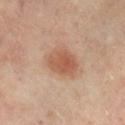This lesion was catalogued during total-body skin photography and was not selected for biopsy. A 15 mm close-up extracted from a 3D total-body photography capture. The lesion is on the leg. An algorithmic analysis of the crop reported an eccentricity of roughly 0.6 and two-axis asymmetry of about 0.15. It also reported border irregularity of about 2 on a 0–10 scale, internal color variation of about 3.5 on a 0–10 scale, and peripheral color asymmetry of about 1. The tile uses cross-polarized illumination. The lesion's longest dimension is about 4 mm. A male patient approximately 55 years of age.